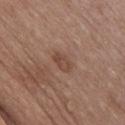Imaged during a routine full-body skin examination; the lesion was not biopsied and no histopathology is available. The lesion is located on the chest. The recorded lesion diameter is about 3 mm. A male patient, aged 48 to 52. A 15 mm close-up extracted from a 3D total-body photography capture. Automated tile analysis of the lesion measured peripheral color asymmetry of about 1. It also reported lesion-presence confidence of about 100/100.